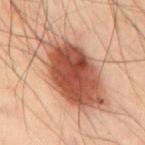Assessment:
Part of a total-body skin-imaging series; this lesion was reviewed on a skin check and was not flagged for biopsy.
Context:
The recorded lesion diameter is about 10.5 mm. This image is a 15 mm lesion crop taken from a total-body photograph. This is a cross-polarized tile. The subject is a male roughly 50 years of age. On the mid back. The total-body-photography lesion software estimated border irregularity of about 3 on a 0–10 scale and a color-variation rating of about 9/10. It also reported a nevus-likeness score of about 95/100 and lesion-presence confidence of about 100/100.